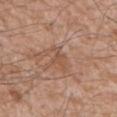Notes:
- follow-up — total-body-photography surveillance lesion; no biopsy
- subject — male, aged 58 to 62
- acquisition — 15 mm crop, total-body photography
- anatomic site — the lower back
- image-analysis metrics — a lesion area of about 3 mm² and an eccentricity of roughly 0.8; an automated nevus-likeness rating near 0 out of 100 and a lesion-detection confidence of about 80/100
- lesion size — ≈2.5 mm